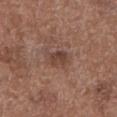This lesion was catalogued during total-body skin photography and was not selected for biopsy. The lesion's longest dimension is about 2.5 mm. Captured under white-light illumination. Located on the left lower leg. The subject is a male in their mid-70s. A 15 mm crop from a total-body photograph taken for skin-cancer surveillance.